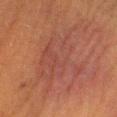Q: Is there a histopathology result?
A: imaged on a skin check; not biopsied
Q: Who is the patient?
A: female, about 40 years old
Q: Where on the body is the lesion?
A: the right lower leg
Q: Automated lesion metrics?
A: an area of roughly 29 mm², an eccentricity of roughly 0.85, and two-axis asymmetry of about 0.45; a lesion-detection confidence of about 90/100
Q: What is the imaging modality?
A: ~15 mm crop, total-body skin-cancer survey
Q: Lesion size?
A: ≈11 mm